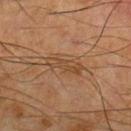Impression:
Part of a total-body skin-imaging series; this lesion was reviewed on a skin check and was not flagged for biopsy.
Image and clinical context:
On the front of the torso. Longest diameter approximately 4.5 mm. Imaged with cross-polarized lighting. Automated image analysis of the tile measured a footprint of about 6 mm², a shape eccentricity near 0.95, and two-axis asymmetry of about 0.25. The software also gave a mean CIELAB color near L≈39 a*≈17 b*≈29 and about 6 CIELAB-L* units darker than the surrounding skin. And it measured a border-irregularity index near 3.5/10 and a peripheral color-asymmetry measure near 1. A male subject roughly 65 years of age. A 15 mm close-up extracted from a 3D total-body photography capture.